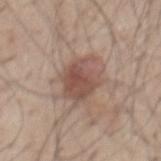Notes:
- biopsy status · catalogued during a skin exam; not biopsied
- TBP lesion metrics · a mean CIELAB color near L≈51 a*≈18 b*≈24, roughly 11 lightness units darker than nearby skin, and a normalized border contrast of about 8; a nevus-likeness score of about 80/100
- lesion diameter · ≈5 mm
- image source · ~15 mm tile from a whole-body skin photo
- subject · male, aged around 50
- location · the mid back